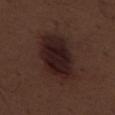Impression:
No biopsy was performed on this lesion — it was imaged during a full skin examination and was not determined to be concerning.
Acquisition and patient details:
The total-body-photography lesion software estimated a within-lesion color-variation index near 4.5/10 and peripheral color asymmetry of about 1.5. The software also gave an automated nevus-likeness rating near 40 out of 100. A close-up tile cropped from a whole-body skin photograph, about 15 mm across. A male subject aged around 70. The lesion is on the left thigh. Measured at roughly 7.5 mm in maximum diameter.